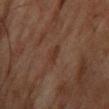The lesion was photographed on a routine skin check and not biopsied; there is no pathology result.
Located on the chest.
The tile uses cross-polarized illumination.
A male subject, roughly 75 years of age.
Measured at roughly 3 mm in maximum diameter.
A region of skin cropped from a whole-body photographic capture, roughly 15 mm wide.
The lesion-visualizer software estimated an average lesion color of about L≈27 a*≈17 b*≈23 (CIELAB), a lesion–skin lightness drop of about 5, and a normalized lesion–skin contrast near 6. It also reported a border-irregularity rating of about 3.5/10, internal color variation of about 0 on a 0–10 scale, and radial color variation of about 0. And it measured a nevus-likeness score of about 0/100 and lesion-presence confidence of about 95/100.